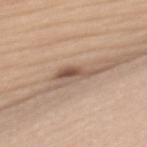Impression:
Captured during whole-body skin photography for melanoma surveillance; the lesion was not biopsied.
Clinical summary:
From the mid back. Imaged with white-light lighting. Cropped from a total-body skin-imaging series; the visible field is about 15 mm. Longest diameter approximately 4 mm. A female subject about 45 years old. The total-body-photography lesion software estimated a shape eccentricity near 0.95 and a shape-asymmetry score of about 0.45 (0 = symmetric). The software also gave a mean CIELAB color near L≈53 a*≈17 b*≈27, about 12 CIELAB-L* units darker than the surrounding skin, and a normalized border contrast of about 8.5.